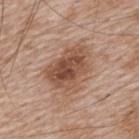Recorded during total-body skin imaging; not selected for excision or biopsy. Located on the upper back. A roughly 15 mm field-of-view crop from a total-body skin photograph. A male patient, aged 63 to 67.